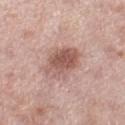biopsy_status: not biopsied; imaged during a skin examination